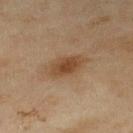Q: Is there a histopathology result?
A: total-body-photography surveillance lesion; no biopsy
Q: Lesion size?
A: about 4 mm
Q: What did automated image analysis measure?
A: a lesion area of about 7 mm², an outline eccentricity of about 0.85 (0 = round, 1 = elongated), and a symmetry-axis asymmetry near 0.15; a mean CIELAB color near L≈41 a*≈17 b*≈30, a lesion–skin lightness drop of about 9, and a normalized border contrast of about 8; border irregularity of about 2 on a 0–10 scale, internal color variation of about 3 on a 0–10 scale, and radial color variation of about 0.5; a nevus-likeness score of about 70/100 and lesion-presence confidence of about 100/100
Q: How was the tile lit?
A: cross-polarized illumination
Q: What is the imaging modality?
A: ~15 mm tile from a whole-body skin photo
Q: Where on the body is the lesion?
A: the leg
Q: Who is the patient?
A: female, in their 60s